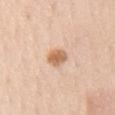| key | value |
|---|---|
| workup | total-body-photography surveillance lesion; no biopsy |
| patient | male, aged around 70 |
| imaging modality | ~15 mm tile from a whole-body skin photo |
| tile lighting | white-light illumination |
| diameter | ≈2.5 mm |
| image-analysis metrics | a footprint of about 4.5 mm², an outline eccentricity of about 0.5 (0 = round, 1 = elongated), and a symmetry-axis asymmetry near 0.15; a color-variation rating of about 3.5/10 and peripheral color asymmetry of about 1.5; a lesion-detection confidence of about 100/100 |
| location | the front of the torso |